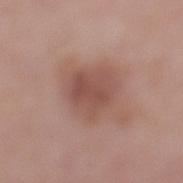Impression: No biopsy was performed on this lesion — it was imaged during a full skin examination and was not determined to be concerning. Image and clinical context: A female patient, aged around 40. On the left lower leg. A 15 mm crop from a total-body photograph taken for skin-cancer surveillance. Measured at roughly 5.5 mm in maximum diameter.